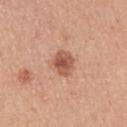Assessment:
Recorded during total-body skin imaging; not selected for excision or biopsy.